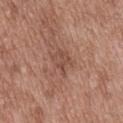Q: Is there a histopathology result?
A: catalogued during a skin exam; not biopsied
Q: Where on the body is the lesion?
A: the mid back
Q: Automated lesion metrics?
A: a footprint of about 6 mm², an eccentricity of roughly 0.75, and a shape-asymmetry score of about 0.3 (0 = symmetric); an automated nevus-likeness rating near 0 out of 100 and a lesion-detection confidence of about 100/100
Q: What are the patient's age and sex?
A: male, approximately 50 years of age
Q: How large is the lesion?
A: ~4 mm (longest diameter)
Q: What kind of image is this?
A: 15 mm crop, total-body photography